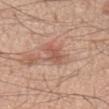This lesion was catalogued during total-body skin photography and was not selected for biopsy. A male patient aged approximately 75. A 15 mm crop from a total-body photograph taken for skin-cancer surveillance. Longest diameter approximately 4 mm. This is a white-light tile. On the chest.